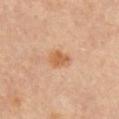Assessment: The lesion was photographed on a routine skin check and not biopsied; there is no pathology result. Clinical summary: A male patient aged around 65. Measured at roughly 3 mm in maximum diameter. Cropped from a total-body skin-imaging series; the visible field is about 15 mm. From the chest. Imaged with cross-polarized lighting. An algorithmic analysis of the crop reported a footprint of about 5 mm², an eccentricity of roughly 0.75, and two-axis asymmetry of about 0.25.